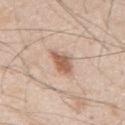Q: Is there a histopathology result?
A: catalogued during a skin exam; not biopsied
Q: Lesion size?
A: ~4 mm (longest diameter)
Q: What is the anatomic site?
A: the abdomen
Q: How was this image acquired?
A: ~15 mm crop, total-body skin-cancer survey
Q: What lighting was used for the tile?
A: white-light
Q: Who is the patient?
A: male, aged around 45